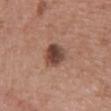This lesion was catalogued during total-body skin photography and was not selected for biopsy.
From the chest.
The patient is a female aged 43–47.
The recorded lesion diameter is about 3.5 mm.
Cropped from a whole-body photographic skin survey; the tile spans about 15 mm.